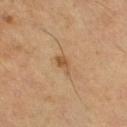The lesion was tiled from a total-body skin photograph and was not biopsied.
The lesion's longest dimension is about 2.5 mm.
The lesion is on the right lower leg.
A 15 mm crop from a total-body photograph taken for skin-cancer surveillance.
A female patient aged approximately 55.
This is a cross-polarized tile.